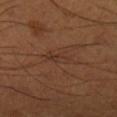The lesion was tiled from a total-body skin photograph and was not biopsied.
An algorithmic analysis of the crop reported an area of roughly 4.5 mm². And it measured an average lesion color of about L≈33 a*≈18 b*≈26 (CIELAB) and about 5 CIELAB-L* units darker than the surrounding skin. And it measured an automated nevus-likeness rating near 0 out of 100.
A roughly 15 mm field-of-view crop from a total-body skin photograph.
Captured under cross-polarized illumination.
A male patient, aged around 50.
The lesion is located on the right lower leg.
The recorded lesion diameter is about 3.5 mm.